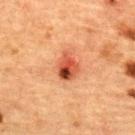follow-up=no biopsy performed (imaged during a skin exam) | subject=female, aged 68 to 72 | anatomic site=the upper back | acquisition=15 mm crop, total-body photography | lighting=cross-polarized.